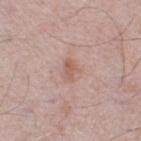follow-up: total-body-photography surveillance lesion; no biopsy | diameter: ≈2.5 mm | body site: the left thigh | subject: male, in their mid-70s | illumination: white-light illumination | image source: 15 mm crop, total-body photography.